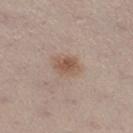follow-up: no biopsy performed (imaged during a skin exam) | site: the left lower leg | size: ~3 mm (longest diameter) | illumination: white-light illumination | automated metrics: a lesion color around L≈54 a*≈17 b*≈27 in CIELAB and about 9 CIELAB-L* units darker than the surrounding skin; a within-lesion color-variation index near 3/10 and radial color variation of about 1 | subject: female, aged 38 to 42 | image: 15 mm crop, total-body photography.